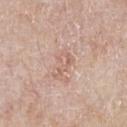<lesion>
  <biopsy_status>not biopsied; imaged during a skin examination</biopsy_status>
  <lighting>white-light</lighting>
  <automated_metrics>
    <cielab_L>62</cielab_L>
    <cielab_a>20</cielab_a>
    <cielab_b>27</cielab_b>
    <vs_skin_darker_L>7.0</vs_skin_darker_L>
    <vs_skin_contrast_norm>5.0</vs_skin_contrast_norm>
    <border_irregularity_0_10>6.5</border_irregularity_0_10>
    <color_variation_0_10>3.5</color_variation_0_10>
    <peripheral_color_asymmetry>1.5</peripheral_color_asymmetry>
    <nevus_likeness_0_100>0</nevus_likeness_0_100>
  </automated_metrics>
  <patient>
    <sex>male</sex>
    <age_approx>65</age_approx>
  </patient>
  <image>
    <source>total-body photography crop</source>
    <field_of_view_mm>15</field_of_view_mm>
  </image>
  <site>chest</site>
</lesion>